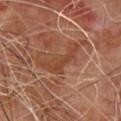Impression: No biopsy was performed on this lesion — it was imaged during a full skin examination and was not determined to be concerning. Image and clinical context: This image is a 15 mm lesion crop taken from a total-body photograph. A male subject, aged 63–67. Approximately 5.5 mm at its widest. Automated tile analysis of the lesion measured a footprint of about 7 mm², an eccentricity of roughly 0.95, and a shape-asymmetry score of about 0.65 (0 = symmetric). It also reported a border-irregularity index near 9/10, a within-lesion color-variation index near 1.5/10, and peripheral color asymmetry of about 0.5.